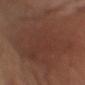Impression: No biopsy was performed on this lesion — it was imaged during a full skin examination and was not determined to be concerning. Clinical summary: An algorithmic analysis of the crop reported a within-lesion color-variation index near 4/10 and a peripheral color-asymmetry measure near 1.5. And it measured lesion-presence confidence of about 70/100. The lesion's longest dimension is about 13.5 mm. The subject is a male approximately 75 years of age. The lesion is located on the left upper arm. Imaged with white-light lighting. A roughly 15 mm field-of-view crop from a total-body skin photograph.